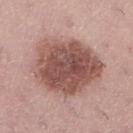  biopsy_status: not biopsied; imaged during a skin examination
  patient:
    sex: female
    age_approx: 40
  site: right lower leg
  lesion_size:
    long_diameter_mm_approx: 8.5
  automated_metrics:
    area_mm2_approx: 40.0
    cielab_L: 52
    cielab_a: 22
    cielab_b: 23
    vs_skin_darker_L: 15.0
  image:
    source: total-body photography crop
    field_of_view_mm: 15